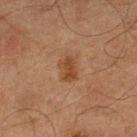This lesion was catalogued during total-body skin photography and was not selected for biopsy.
The subject is a male in their mid-60s.
On the right lower leg.
This image is a 15 mm lesion crop taken from a total-body photograph.